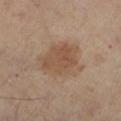- workup — imaged on a skin check; not biopsied
- subject — female, in their 60s
- body site — the left lower leg
- tile lighting — cross-polarized
- image-analysis metrics — a footprint of about 19 mm², an outline eccentricity of about 0.6 (0 = round, 1 = elongated), and a shape-asymmetry score of about 0.3 (0 = symmetric); an automated nevus-likeness rating near 45 out of 100
- image — 15 mm crop, total-body photography